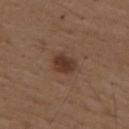The lesion was photographed on a routine skin check and not biopsied; there is no pathology result.
From the mid back.
The subject is a male in their mid-70s.
A close-up tile cropped from a whole-body skin photograph, about 15 mm across.